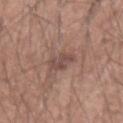| key | value |
|---|---|
| workup | total-body-photography surveillance lesion; no biopsy |
| acquisition | total-body-photography crop, ~15 mm field of view |
| TBP lesion metrics | a mean CIELAB color near L≈48 a*≈18 b*≈22, roughly 8 lightness units darker than nearby skin, and a normalized lesion–skin contrast near 6.5; a border-irregularity rating of about 3/10, a within-lesion color-variation index near 3/10, and a peripheral color-asymmetry measure near 1 |
| diameter | ~3.5 mm (longest diameter) |
| patient | male, aged 53 to 57 |
| illumination | white-light illumination |